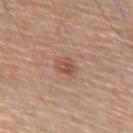The lesion was tiled from a total-body skin photograph and was not biopsied. A male patient about 60 years old. A close-up tile cropped from a whole-body skin photograph, about 15 mm across. Measured at roughly 2.5 mm in maximum diameter. The tile uses white-light illumination. Located on the right upper arm.